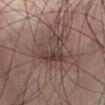Recorded during total-body skin imaging; not selected for excision or biopsy. A 15 mm crop from a total-body photograph taken for skin-cancer surveillance. Approximately 4.5 mm at its widest. The total-body-photography lesion software estimated an average lesion color of about L≈35 a*≈14 b*≈17 (CIELAB), about 7 CIELAB-L* units darker than the surrounding skin, and a normalized lesion–skin contrast near 7. The lesion is on the front of the torso. A male subject about 50 years old.